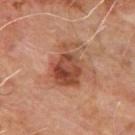Findings:
- biopsy status: total-body-photography surveillance lesion; no biopsy
- subject: male, aged 63 to 67
- TBP lesion metrics: a shape eccentricity near 0.7 and a shape-asymmetry score of about 0.35 (0 = symmetric); an average lesion color of about L≈45 a*≈24 b*≈31 (CIELAB) and a lesion–skin lightness drop of about 12; border irregularity of about 5.5 on a 0–10 scale, a color-variation rating of about 6.5/10, and radial color variation of about 2; a classifier nevus-likeness of about 55/100 and lesion-presence confidence of about 100/100
- imaging modality: ~15 mm crop, total-body skin-cancer survey
- lesion size: ~5.5 mm (longest diameter)
- body site: the upper back
- tile lighting: cross-polarized